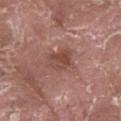  biopsy_status: not biopsied; imaged during a skin examination
  image:
    source: total-body photography crop
    field_of_view_mm: 15
  automated_metrics:
    cielab_L: 46
    cielab_a: 22
    cielab_b: 25
    nevus_likeness_0_100: 0
    lesion_detection_confidence_0_100: 100
  patient:
    sex: male
    age_approx: 40
  site: right thigh
  lighting: white-light